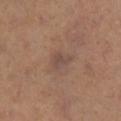{
  "biopsy_status": "not biopsied; imaged during a skin examination",
  "lighting": "cross-polarized",
  "image": {
    "source": "total-body photography crop",
    "field_of_view_mm": 15
  },
  "lesion_size": {
    "long_diameter_mm_approx": 2.5
  },
  "patient": {
    "sex": "female",
    "age_approx": 40
  },
  "site": "right lower leg"
}